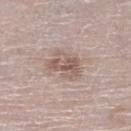This lesion was catalogued during total-body skin photography and was not selected for biopsy. This image is a 15 mm lesion crop taken from a total-body photograph. Longest diameter approximately 4.5 mm. The lesion is on the right lower leg. A male subject, aged 78 to 82. Captured under white-light illumination.